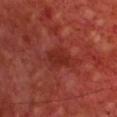Part of a total-body skin-imaging series; this lesion was reviewed on a skin check and was not flagged for biopsy. A male subject, aged approximately 70. The lesion is on the chest. This image is a 15 mm lesion crop taken from a total-body photograph. The total-body-photography lesion software estimated a shape eccentricity near 0.7 and a shape-asymmetry score of about 0.25 (0 = symmetric). The analysis additionally found a nevus-likeness score of about 0/100 and lesion-presence confidence of about 90/100. Captured under cross-polarized illumination.